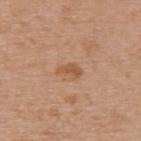Captured during whole-body skin photography for melanoma surveillance; the lesion was not biopsied.
The lesion-visualizer software estimated about 9 CIELAB-L* units darker than the surrounding skin and a lesion-to-skin contrast of about 6.5 (normalized; higher = more distinct). The analysis additionally found a classifier nevus-likeness of about 10/100 and lesion-presence confidence of about 100/100.
The recorded lesion diameter is about 2.5 mm.
On the back.
A female patient aged around 65.
This is a white-light tile.
A 15 mm close-up tile from a total-body photography series done for melanoma screening.